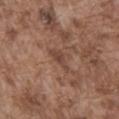• biopsy status — no biopsy performed (imaged during a skin exam)
• lesion diameter — ≈3.5 mm
• tile lighting — white-light illumination
• patient — male, approximately 75 years of age
• imaging modality — 15 mm crop, total-body photography
• site — the abdomen